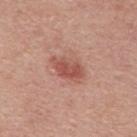Captured during whole-body skin photography for melanoma surveillance; the lesion was not biopsied.
An algorithmic analysis of the crop reported an eccentricity of roughly 0.8 and two-axis asymmetry of about 0.2. The software also gave about 10 CIELAB-L* units darker than the surrounding skin. And it measured a nevus-likeness score of about 75/100 and a detector confidence of about 100 out of 100 that the crop contains a lesion.
Imaged with white-light lighting.
This image is a 15 mm lesion crop taken from a total-body photograph.
The subject is a male approximately 60 years of age.
About 4 mm across.
Located on the mid back.